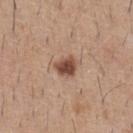Recorded during total-body skin imaging; not selected for excision or biopsy. The recorded lesion diameter is about 2.5 mm. The subject is a male aged around 60. Imaged with white-light lighting. A 15 mm close-up extracted from a 3D total-body photography capture. The lesion is on the front of the torso.